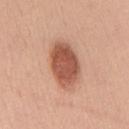Findings:
• notes — total-body-photography surveillance lesion; no biopsy